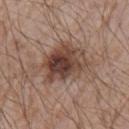Assessment: Captured during whole-body skin photography for melanoma surveillance; the lesion was not biopsied. Background: The total-body-photography lesion software estimated a border-irregularity rating of about 5/10 and a within-lesion color-variation index near 8.5/10. It also reported an automated nevus-likeness rating near 90 out of 100 and a lesion-detection confidence of about 100/100. The subject is a male aged approximately 75. Approximately 7 mm at its widest. This is a white-light tile. The lesion is on the arm. A roughly 15 mm field-of-view crop from a total-body skin photograph.